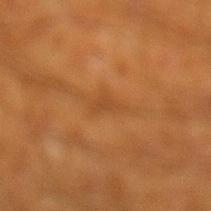– notes: catalogued during a skin exam; not biopsied
– image: ~15 mm crop, total-body skin-cancer survey
– body site: the left lower leg
– patient: male, approximately 60 years of age
– automated metrics: an average lesion color of about L≈38 a*≈21 b*≈35 (CIELAB), a lesion–skin lightness drop of about 5, and a normalized lesion–skin contrast near 4.5; an automated nevus-likeness rating near 0 out of 100 and a lesion-detection confidence of about 95/100
– illumination: cross-polarized